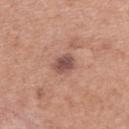Impression:
The lesion was photographed on a routine skin check and not biopsied; there is no pathology result.
Context:
A 15 mm close-up tile from a total-body photography series done for melanoma screening. Located on the upper back. A female subject, approximately 60 years of age.